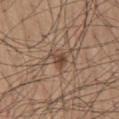The lesion was tiled from a total-body skin photograph and was not biopsied.
The lesion is on the mid back.
Cropped from a whole-body photographic skin survey; the tile spans about 15 mm.
A male subject, in their mid- to late 60s.